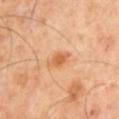Imaged during a routine full-body skin examination; the lesion was not biopsied and no histopathology is available. The patient is a male aged 63 to 67. The lesion-visualizer software estimated a lesion area of about 4 mm², an outline eccentricity of about 0.75 (0 = round, 1 = elongated), and a symmetry-axis asymmetry near 0.2. It also reported an average lesion color of about L≈61 a*≈25 b*≈40 (CIELAB), a lesion–skin lightness drop of about 10, and a lesion-to-skin contrast of about 7 (normalized; higher = more distinct). And it measured a detector confidence of about 100 out of 100 that the crop contains a lesion. A 15 mm close-up extracted from a 3D total-body photography capture. This is a cross-polarized tile.